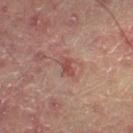Part of a total-body skin-imaging series; this lesion was reviewed on a skin check and was not flagged for biopsy. This is a cross-polarized tile. The lesion-visualizer software estimated an area of roughly 3.5 mm², an outline eccentricity of about 0.85 (0 = round, 1 = elongated), and a symmetry-axis asymmetry near 0.35. And it measured an average lesion color of about L≈43 a*≈23 b*≈21 (CIELAB), roughly 8 lightness units darker than nearby skin, and a lesion-to-skin contrast of about 6.5 (normalized; higher = more distinct). And it measured a border-irregularity rating of about 3.5/10 and a color-variation rating of about 1/10. A male patient, approximately 60 years of age. Cropped from a total-body skin-imaging series; the visible field is about 15 mm. From the right lower leg.